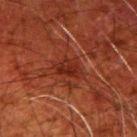No biopsy was performed on this lesion — it was imaged during a full skin examination and was not determined to be concerning. A male subject aged approximately 80. A roughly 15 mm field-of-view crop from a total-body skin photograph. Measured at roughly 3.5 mm in maximum diameter. On the arm.